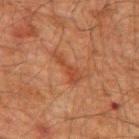Recorded during total-body skin imaging; not selected for excision or biopsy. A lesion tile, about 15 mm wide, cut from a 3D total-body photograph. A male subject aged 58–62. Automated tile analysis of the lesion measured a footprint of about 4.5 mm² and two-axis asymmetry of about 0.6. And it measured roughly 6 lightness units darker than nearby skin and a normalized lesion–skin contrast near 6. And it measured a lesion-detection confidence of about 100/100. The recorded lesion diameter is about 4 mm. Located on the mid back.